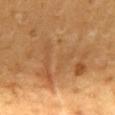Q: Is there a histopathology result?
A: total-body-photography surveillance lesion; no biopsy
Q: How was this image acquired?
A: 15 mm crop, total-body photography
Q: Automated lesion metrics?
A: a footprint of about 32 mm², a shape eccentricity near 0.8, and a symmetry-axis asymmetry near 0.45; a mean CIELAB color near L≈52 a*≈20 b*≈38, a lesion–skin lightness drop of about 7, and a lesion-to-skin contrast of about 5 (normalized; higher = more distinct)
Q: What are the patient's age and sex?
A: male, about 60 years old
Q: What is the anatomic site?
A: the mid back